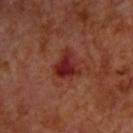No biopsy was performed on this lesion — it was imaged during a full skin examination and was not determined to be concerning.
An algorithmic analysis of the crop reported a mean CIELAB color near L≈28 a*≈30 b*≈25, a lesion–skin lightness drop of about 9, and a lesion-to-skin contrast of about 9.5 (normalized; higher = more distinct). It also reported a classifier nevus-likeness of about 0/100 and a detector confidence of about 100 out of 100 that the crop contains a lesion.
About 3.5 mm across.
This is a cross-polarized tile.
A lesion tile, about 15 mm wide, cut from a 3D total-body photograph.
A male patient, roughly 70 years of age.
The lesion is located on the upper back.